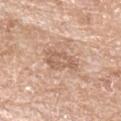Recorded during total-body skin imaging; not selected for excision or biopsy. A female subject, aged 73 to 77. This image is a 15 mm lesion crop taken from a total-body photograph. From the left forearm.